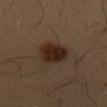Assessment:
The lesion was tiled from a total-body skin photograph and was not biopsied.
Acquisition and patient details:
The lesion is located on the chest. A male subject, aged around 55. The lesion's longest dimension is about 3.5 mm. Captured under cross-polarized illumination. A close-up tile cropped from a whole-body skin photograph, about 15 mm across. The lesion-visualizer software estimated an area of roughly 10 mm², an outline eccentricity of about 0.4 (0 = round, 1 = elongated), and two-axis asymmetry of about 0.2.